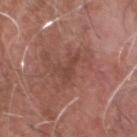- workup — imaged on a skin check; not biopsied
- subject — male, about 75 years old
- body site — the head or neck
- lesion size — ≈3.5 mm
- acquisition — 15 mm crop, total-body photography